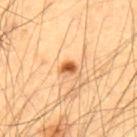No biopsy was performed on this lesion — it was imaged during a full skin examination and was not determined to be concerning. From the upper back. The patient is a male approximately 55 years of age. Cropped from a total-body skin-imaging series; the visible field is about 15 mm.